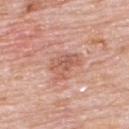patient:
  sex: male
  age_approx: 80
lighting: white-light
site: upper back
lesion_size:
  long_diameter_mm_approx: 3.5
image:
  source: total-body photography crop
  field_of_view_mm: 15
automated_metrics:
  cielab_L: 58
  cielab_a: 25
  cielab_b: 29
  vs_skin_darker_L: 9.0
  border_irregularity_0_10: 5.0
  color_variation_0_10: 3.0